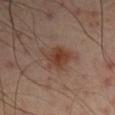Captured during whole-body skin photography for melanoma surveillance; the lesion was not biopsied. On the left arm. Cropped from a total-body skin-imaging series; the visible field is about 15 mm. The patient is a male aged approximately 50. The recorded lesion diameter is about 4.5 mm. An algorithmic analysis of the crop reported border irregularity of about 4.5 on a 0–10 scale, a within-lesion color-variation index near 4/10, and radial color variation of about 1. This is a cross-polarized tile.